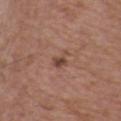Recorded during total-body skin imaging; not selected for excision or biopsy.
Approximately 2.5 mm at its widest.
The lesion is on the back.
A 15 mm crop from a total-body photograph taken for skin-cancer surveillance.
A male subject, roughly 50 years of age.